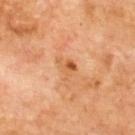Part of a total-body skin-imaging series; this lesion was reviewed on a skin check and was not flagged for biopsy.
Automated tile analysis of the lesion measured roughly 9 lightness units darker than nearby skin and a normalized lesion–skin contrast near 6.5.
This image is a 15 mm lesion crop taken from a total-body photograph.
Imaged with cross-polarized lighting.
Approximately 2.5 mm at its widest.
The patient is a male aged 68–72.
The lesion is located on the back.